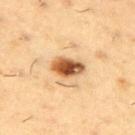Clinical impression: The lesion was tiled from a total-body skin photograph and was not biopsied. Clinical summary: A male patient roughly 55 years of age. The lesion is located on the right upper arm. A 15 mm close-up extracted from a 3D total-body photography capture. Longest diameter approximately 4 mm.